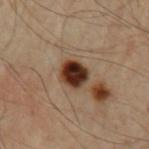Q: Is there a histopathology result?
A: total-body-photography surveillance lesion; no biopsy
Q: Lesion location?
A: the left upper arm
Q: What are the patient's age and sex?
A: male, aged approximately 65
Q: How was this image acquired?
A: ~15 mm crop, total-body skin-cancer survey